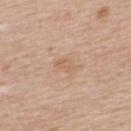Imaged during a routine full-body skin examination; the lesion was not biopsied and no histopathology is available. Measured at roughly 2.5 mm in maximum diameter. On the upper back. The patient is a female aged approximately 45. Cropped from a whole-body photographic skin survey; the tile spans about 15 mm. Automated image analysis of the tile measured an eccentricity of roughly 0.8. And it measured a lesion color around L≈62 a*≈18 b*≈33 in CIELAB, a lesion–skin lightness drop of about 6, and a normalized lesion–skin contrast near 5. The analysis additionally found a classifier nevus-likeness of about 0/100 and lesion-presence confidence of about 100/100. The tile uses white-light illumination.